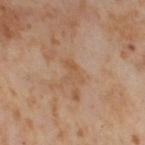Captured during whole-body skin photography for melanoma surveillance; the lesion was not biopsied.
A female subject, roughly 55 years of age.
On the right thigh.
Measured at roughly 3 mm in maximum diameter.
The lesion-visualizer software estimated an eccentricity of roughly 0.8 and two-axis asymmetry of about 0.45. And it measured lesion-presence confidence of about 100/100.
A region of skin cropped from a whole-body photographic capture, roughly 15 mm wide.
Captured under cross-polarized illumination.